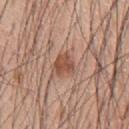Assessment: The lesion was photographed on a routine skin check and not biopsied; there is no pathology result. Clinical summary: A 15 mm close-up extracted from a 3D total-body photography capture. The patient is a male about 60 years old. The lesion is located on the chest. The recorded lesion diameter is about 3 mm.